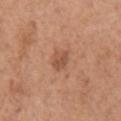This lesion was catalogued during total-body skin photography and was not selected for biopsy.
A female subject, aged around 65.
Cropped from a total-body skin-imaging series; the visible field is about 15 mm.
An algorithmic analysis of the crop reported an area of roughly 4 mm², a shape eccentricity near 0.7, and a symmetry-axis asymmetry near 0.25. The software also gave a lesion color around L≈52 a*≈23 b*≈32 in CIELAB, roughly 9 lightness units darker than nearby skin, and a normalized border contrast of about 6.5. The software also gave internal color variation of about 1.5 on a 0–10 scale.
From the left upper arm.
Longest diameter approximately 3 mm.